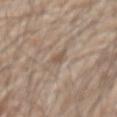Findings:
– patient · male, roughly 80 years of age
– acquisition · ~15 mm crop, total-body skin-cancer survey
– site · the abdomen
– automated metrics · a footprint of about 2.5 mm², a shape eccentricity near 0.85, and a symmetry-axis asymmetry near 0.4; border irregularity of about 4 on a 0–10 scale and a color-variation rating of about 1/10; a detector confidence of about 70 out of 100 that the crop contains a lesion
– tile lighting · white-light
– lesion size · ≈2.5 mm